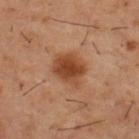This lesion was catalogued during total-body skin photography and was not selected for biopsy.
On the upper back.
A male subject, in their mid- to late 50s.
The total-body-photography lesion software estimated two-axis asymmetry of about 0.2. The software also gave an average lesion color of about L≈43 a*≈24 b*≈34 (CIELAB), roughly 12 lightness units darker than nearby skin, and a normalized border contrast of about 9.5.
Measured at roughly 4 mm in maximum diameter.
A close-up tile cropped from a whole-body skin photograph, about 15 mm across.